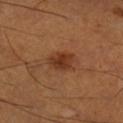{"biopsy_status": "not biopsied; imaged during a skin examination", "patient": {"sex": "male", "age_approx": 50}, "lighting": "cross-polarized", "site": "leg", "image": {"source": "total-body photography crop", "field_of_view_mm": 15}, "lesion_size": {"long_diameter_mm_approx": 3.5}}